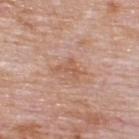Q: Where on the body is the lesion?
A: the upper back
Q: How was this image acquired?
A: 15 mm crop, total-body photography
Q: Patient demographics?
A: male, about 75 years old